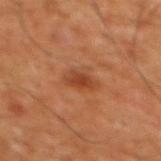| field | value |
|---|---|
| workup | total-body-photography surveillance lesion; no biopsy |
| image-analysis metrics | a footprint of about 4.5 mm², an outline eccentricity of about 0.85 (0 = round, 1 = elongated), and a symmetry-axis asymmetry near 0.25; an average lesion color of about L≈39 a*≈26 b*≈34 (CIELAB) and roughly 8 lightness units darker than nearby skin |
| lesion size | about 3 mm |
| tile lighting | cross-polarized |
| patient | male, aged around 60 |
| location | the chest |
| acquisition | ~15 mm tile from a whole-body skin photo |